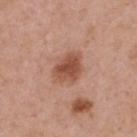| feature | finding |
|---|---|
| biopsy status | total-body-photography surveillance lesion; no biopsy |
| patient | male, in their mid-50s |
| illumination | white-light illumination |
| acquisition | ~15 mm crop, total-body skin-cancer survey |
| image-analysis metrics | border irregularity of about 2.5 on a 0–10 scale and a peripheral color-asymmetry measure near 1 |
| location | the upper back |
| diameter | ≈4 mm |